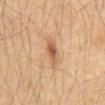The tile uses cross-polarized illumination. From the abdomen. Automated image analysis of the tile measured an area of roughly 4.5 mm², a shape eccentricity near 0.85, and two-axis asymmetry of about 0.25. The analysis additionally found an average lesion color of about L≈43 a*≈17 b*≈28 (CIELAB), about 9 CIELAB-L* units darker than the surrounding skin, and a normalized lesion–skin contrast near 7. The software also gave a border-irregularity index near 2.5/10. Measured at roughly 3 mm in maximum diameter. A male patient, aged 58 to 62. A 15 mm close-up tile from a total-body photography series done for melanoma screening.